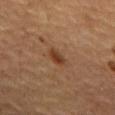Recorded during total-body skin imaging; not selected for excision or biopsy. Imaged with cross-polarized lighting. A region of skin cropped from a whole-body photographic capture, roughly 15 mm wide. Automated tile analysis of the lesion measured a lesion area of about 3.5 mm², an eccentricity of roughly 0.8, and a shape-asymmetry score of about 0.3 (0 = symmetric). It also reported a lesion color around L≈34 a*≈19 b*≈29 in CIELAB, about 9 CIELAB-L* units darker than the surrounding skin, and a normalized lesion–skin contrast near 8. The analysis additionally found border irregularity of about 3 on a 0–10 scale, internal color variation of about 2.5 on a 0–10 scale, and radial color variation of about 1. And it measured lesion-presence confidence of about 100/100. Longest diameter approximately 2.5 mm. On the chest. The subject is a male about 65 years old.